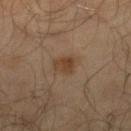<tbp_lesion>
  <biopsy_status>not biopsied; imaged during a skin examination</biopsy_status>
  <site>right lower leg</site>
  <patient>
    <sex>male</sex>
    <age_approx>65</age_approx>
  </patient>
  <image>
    <source>total-body photography crop</source>
    <field_of_view_mm>15</field_of_view_mm>
  </image>
  <lesion_size>
    <long_diameter_mm_approx>3.0</long_diameter_mm_approx>
  </lesion_size>
</tbp_lesion>